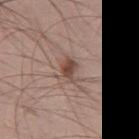No biopsy was performed on this lesion — it was imaged during a full skin examination and was not determined to be concerning.